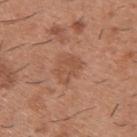  lighting: white-light
  image:
    source: total-body photography crop
    field_of_view_mm: 15
  lesion_size:
    long_diameter_mm_approx: 3.5
  automated_metrics:
    eccentricity: 0.6
    shape_asymmetry: 0.25
    cielab_L: 52
    cielab_a: 23
    cielab_b: 31
    vs_skin_contrast_norm: 5.0
    border_irregularity_0_10: 2.5
    peripheral_color_asymmetry: 1.0
  site: back
  patient:
    sex: male
    age_approx: 40